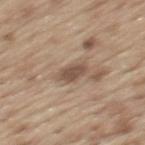Q: Was a biopsy performed?
A: total-body-photography surveillance lesion; no biopsy
Q: What kind of image is this?
A: total-body-photography crop, ~15 mm field of view
Q: What are the patient's age and sex?
A: male, aged 68–72
Q: What is the anatomic site?
A: the upper back